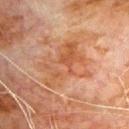location — the front of the torso | acquisition — total-body-photography crop, ~15 mm field of view | patient — male, about 80 years old.